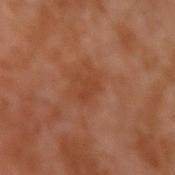follow-up — imaged on a skin check; not biopsied
site — the arm
TBP lesion metrics — a lesion color around L≈41 a*≈25 b*≈33 in CIELAB and a lesion-to-skin contrast of about 5 (normalized; higher = more distinct); radial color variation of about 0; a nevus-likeness score of about 0/100
image source — ~15 mm tile from a whole-body skin photo
subject — male, aged around 30